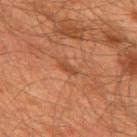Background:
On the upper back. A close-up tile cropped from a whole-body skin photograph, about 15 mm across. A male patient, aged around 60.